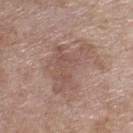A female subject approximately 75 years of age. Imaged with white-light lighting. The lesion is located on the upper back. A close-up tile cropped from a whole-body skin photograph, about 15 mm across. Automated tile analysis of the lesion measured a footprint of about 17 mm² and a shape-asymmetry score of about 0.6 (0 = symmetric). The analysis additionally found a border-irregularity rating of about 7.5/10, a within-lesion color-variation index near 2.5/10, and a peripheral color-asymmetry measure near 1.